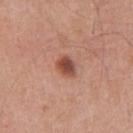{"patient": {"sex": "male", "age_approx": 55}, "image": {"source": "total-body photography crop", "field_of_view_mm": 15}, "lighting": "white-light", "lesion_size": {"long_diameter_mm_approx": 2.5}, "site": "chest"}